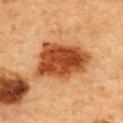Assessment:
Part of a total-body skin-imaging series; this lesion was reviewed on a skin check and was not flagged for biopsy.
Clinical summary:
A female patient aged 58 to 62. Captured under cross-polarized illumination. The lesion is on the back. A roughly 15 mm field-of-view crop from a total-body skin photograph. The total-body-photography lesion software estimated a lesion color around L≈43 a*≈27 b*≈38 in CIELAB, roughly 17 lightness units darker than nearby skin, and a normalized lesion–skin contrast near 12.